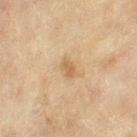Impression:
No biopsy was performed on this lesion — it was imaged during a full skin examination and was not determined to be concerning.
Context:
A female subject, aged 78–82. A region of skin cropped from a whole-body photographic capture, roughly 15 mm wide. Located on the leg. The tile uses cross-polarized illumination. An algorithmic analysis of the crop reported an outline eccentricity of about 0.8 (0 = round, 1 = elongated) and a shape-asymmetry score of about 0.3 (0 = symmetric). The software also gave a border-irregularity rating of about 3/10, a color-variation rating of about 1/10, and peripheral color asymmetry of about 0.5. The software also gave an automated nevus-likeness rating near 10 out of 100 and lesion-presence confidence of about 100/100.